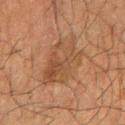The lesion was photographed on a routine skin check and not biopsied; there is no pathology result.
The total-body-photography lesion software estimated a lesion area of about 19 mm², a shape eccentricity near 0.5, and a shape-asymmetry score of about 0.3 (0 = symmetric). And it measured a lesion color around L≈38 a*≈16 b*≈27 in CIELAB, a lesion–skin lightness drop of about 6, and a normalized lesion–skin contrast near 5.5. It also reported a border-irregularity index near 4/10, a color-variation rating of about 4.5/10, and peripheral color asymmetry of about 1.5.
Located on the right forearm.
A male patient aged 58–62.
A region of skin cropped from a whole-body photographic capture, roughly 15 mm wide.